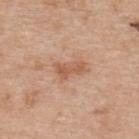biopsy status = catalogued during a skin exam; not biopsied | site = the upper back | image-analysis metrics = a lesion color around L≈58 a*≈22 b*≈33 in CIELAB and a normalized lesion–skin contrast near 6.5; a nevus-likeness score of about 0/100 and lesion-presence confidence of about 100/100 | lesion diameter = about 3.5 mm | acquisition = total-body-photography crop, ~15 mm field of view | subject = male, roughly 70 years of age | tile lighting = white-light.